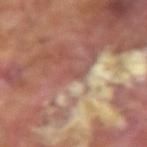Q: Patient demographics?
A: male, in their 70s
Q: What lighting was used for the tile?
A: cross-polarized
Q: How was this image acquired?
A: ~15 mm tile from a whole-body skin photo
Q: What did automated image analysis measure?
A: lesion-presence confidence of about 0/100
Q: Lesion location?
A: the arm